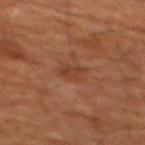Recorded during total-body skin imaging; not selected for excision or biopsy. The tile uses cross-polarized illumination. Approximately 3 mm at its widest. A male patient, aged approximately 60. This image is a 15 mm lesion crop taken from a total-body photograph. The total-body-photography lesion software estimated an area of roughly 5.5 mm² and an eccentricity of roughly 0.45. From the upper back.